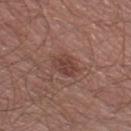notes = imaged on a skin check; not biopsied
subject = male, approximately 55 years of age
imaging modality = ~15 mm tile from a whole-body skin photo
illumination = white-light illumination
body site = the right lower leg
size = ~3 mm (longest diameter)
image-analysis metrics = a lesion area of about 6 mm² and two-axis asymmetry of about 0.3; a border-irregularity rating of about 3/10 and a peripheral color-asymmetry measure near 1